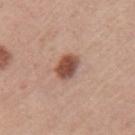Recorded during total-body skin imaging; not selected for excision or biopsy.
Longest diameter approximately 3 mm.
This image is a 15 mm lesion crop taken from a total-body photograph.
On the left upper arm.
A female patient aged 58–62.
Captured under white-light illumination.